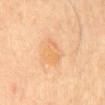Findings:
* notes — catalogued during a skin exam; not biopsied
* patient — aged 63 to 67
* anatomic site — the mid back
* image — ~15 mm tile from a whole-body skin photo
* lesion size — ≈4 mm